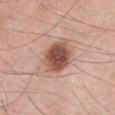Assessment:
No biopsy was performed on this lesion — it was imaged during a full skin examination and was not determined to be concerning.
Context:
The patient is a male about 45 years old. This image is a 15 mm lesion crop taken from a total-body photograph. The lesion is on the leg.